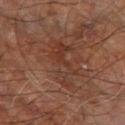notes: no biopsy performed (imaged during a skin exam)
body site: the right leg
patient: male, aged approximately 60
size: about 6.5 mm
lighting: cross-polarized illumination
automated lesion analysis: a lesion area of about 12 mm² and a symmetry-axis asymmetry near 0.45; an average lesion color of about L≈35 a*≈20 b*≈26 (CIELAB), roughly 6 lightness units darker than nearby skin, and a lesion-to-skin contrast of about 5.5 (normalized; higher = more distinct); a nevus-likeness score of about 0/100 and a lesion-detection confidence of about 85/100
image source: ~15 mm crop, total-body skin-cancer survey